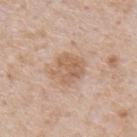The lesion was tiled from a total-body skin photograph and was not biopsied. A roughly 15 mm field-of-view crop from a total-body skin photograph. The patient is a male aged 63–67. Automated tile analysis of the lesion measured a footprint of about 10 mm² and a shape eccentricity near 0.4. It also reported about 9 CIELAB-L* units darker than the surrounding skin. The analysis additionally found border irregularity of about 2.5 on a 0–10 scale. Captured under white-light illumination. Longest diameter approximately 4 mm. Located on the mid back.